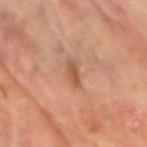The lesion is on the right upper arm.
Measured at roughly 3 mm in maximum diameter.
A 15 mm crop from a total-body photograph taken for skin-cancer surveillance.
A male patient, in their 70s.